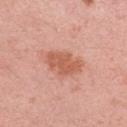Q: Is there a histopathology result?
A: no biopsy performed (imaged during a skin exam)
Q: Automated lesion metrics?
A: a lesion area of about 9 mm², an eccentricity of roughly 0.8, and a shape-asymmetry score of about 0.3 (0 = symmetric); a border-irregularity index near 3.5/10, a color-variation rating of about 2/10, and peripheral color asymmetry of about 0.5; a classifier nevus-likeness of about 55/100 and lesion-presence confidence of about 100/100
Q: Lesion size?
A: ~4.5 mm (longest diameter)
Q: Lesion location?
A: the right upper arm
Q: What lighting was used for the tile?
A: white-light illumination
Q: Who is the patient?
A: female, in their mid-30s
Q: What kind of image is this?
A: 15 mm crop, total-body photography